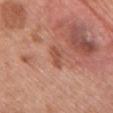image-analysis metrics — an average lesion color of about L≈51 a*≈25 b*≈30 (CIELAB), a lesion–skin lightness drop of about 9, and a normalized border contrast of about 6.5
body site — the chest
patient — female, about 60 years old
illumination — white-light illumination
size — about 3 mm
image — ~15 mm crop, total-body skin-cancer survey